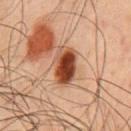No biopsy was performed on this lesion — it was imaged during a full skin examination and was not determined to be concerning. A male patient, aged 43 to 47. Imaged with cross-polarized lighting. Automated image analysis of the tile measured internal color variation of about 10 on a 0–10 scale. Cropped from a whole-body photographic skin survey; the tile spans about 15 mm. The lesion is on the chest. The lesion's longest dimension is about 5 mm.